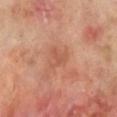Assessment: Captured during whole-body skin photography for melanoma surveillance; the lesion was not biopsied. Background: A male subject, approximately 70 years of age. The lesion is on the left lower leg. About 3 mm across. Automated image analysis of the tile measured an eccentricity of roughly 0.6 and a shape-asymmetry score of about 0.5 (0 = symmetric). The analysis additionally found a border-irregularity index near 5.5/10, a color-variation rating of about 1/10, and peripheral color asymmetry of about 0.5. A region of skin cropped from a whole-body photographic capture, roughly 15 mm wide.